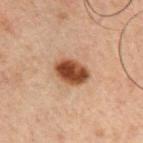{"patient": {"sex": "male", "age_approx": 60}, "site": "chest", "image": {"source": "total-body photography crop", "field_of_view_mm": 15}}